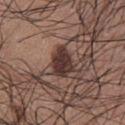  biopsy_status: not biopsied; imaged during a skin examination
  patient:
    sex: male
    age_approx: 35
  site: chest
  lighting: white-light
  image:
    source: total-body photography crop
    field_of_view_mm: 15
  lesion_size:
    long_diameter_mm_approx: 3.5